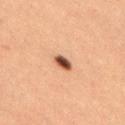Notes:
• biopsy status — catalogued during a skin exam; not biopsied
• body site — the leg
• automated lesion analysis — a footprint of about 3.5 mm², a shape eccentricity near 0.8, and two-axis asymmetry of about 0.25; an average lesion color of about L≈42 a*≈21 b*≈29 (CIELAB); a border-irregularity index near 2/10; lesion-presence confidence of about 100/100
• subject — female, about 40 years old
• imaging modality — total-body-photography crop, ~15 mm field of view
• illumination — cross-polarized